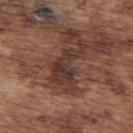Imaged during a routine full-body skin examination; the lesion was not biopsied and no histopathology is available. A male patient, in their mid- to late 70s. Located on the upper back. This image is a 15 mm lesion crop taken from a total-body photograph.